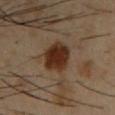workup = no biopsy performed (imaged during a skin exam) | site = the chest | illumination = cross-polarized | subject = male, about 40 years old | size = ~4 mm (longest diameter) | image = total-body-photography crop, ~15 mm field of view.